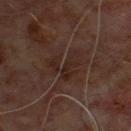automated metrics: a footprint of about 8.5 mm² and an outline eccentricity of about 0.55 (0 = round, 1 = elongated); a border-irregularity index near 9/10, a color-variation rating of about 2.5/10, and radial color variation of about 1; a classifier nevus-likeness of about 0/100 and a lesion-detection confidence of about 95/100 | image source: 15 mm crop, total-body photography | subject: male, aged around 60 | site: the upper back | lesion diameter: ~4 mm (longest diameter) | lighting: cross-polarized.